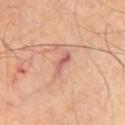This lesion was catalogued during total-body skin photography and was not selected for biopsy.